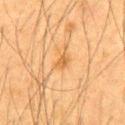Notes:
– notes — no biopsy performed (imaged during a skin exam)
– lighting — cross-polarized illumination
– subject — male, about 35 years old
– size — ~2.5 mm (longest diameter)
– image — ~15 mm crop, total-body skin-cancer survey
– anatomic site — the upper back